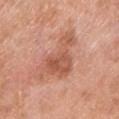No biopsy was performed on this lesion — it was imaged during a full skin examination and was not determined to be concerning. The subject is a male about 80 years old. Located on the left lower leg. Cropped from a total-body skin-imaging series; the visible field is about 15 mm.